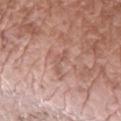The lesion was photographed on a routine skin check and not biopsied; there is no pathology result.
On the left upper arm.
Captured under white-light illumination.
Measured at roughly 3.5 mm in maximum diameter.
A male subject in their mid-50s.
This image is a 15 mm lesion crop taken from a total-body photograph.